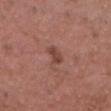This lesion was catalogued during total-body skin photography and was not selected for biopsy.
A close-up tile cropped from a whole-body skin photograph, about 15 mm across.
A male subject aged 48 to 52.
Captured under white-light illumination.
From the head or neck.